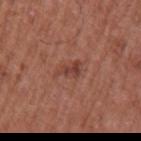{
  "lighting": "white-light",
  "patient": {
    "sex": "male",
    "age_approx": 65
  },
  "image": {
    "source": "total-body photography crop",
    "field_of_view_mm": 15
  },
  "site": "left upper arm"
}